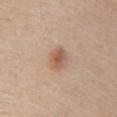Q: Was a biopsy performed?
A: no biopsy performed (imaged during a skin exam)
Q: What is the lesion's diameter?
A: ≈3.5 mm
Q: How was this image acquired?
A: ~15 mm crop, total-body skin-cancer survey
Q: Lesion location?
A: the abdomen
Q: What are the patient's age and sex?
A: male, aged approximately 60
Q: Illumination type?
A: white-light illumination